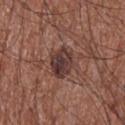<record>
  <biopsy_status>not biopsied; imaged during a skin examination</biopsy_status>
  <automated_metrics>
    <cielab_L>36</cielab_L>
    <cielab_a>19</cielab_a>
    <cielab_b>21</cielab_b>
    <vs_skin_darker_L>11.0</vs_skin_darker_L>
    <vs_skin_contrast_norm>10.0</vs_skin_contrast_norm>
    <lesion_detection_confidence_0_100>100</lesion_detection_confidence_0_100>
  </automated_metrics>
  <patient>
    <sex>male</sex>
    <age_approx>75</age_approx>
  </patient>
  <lighting>white-light</lighting>
  <lesion_size>
    <long_diameter_mm_approx>4.0</long_diameter_mm_approx>
  </lesion_size>
  <image>
    <source>total-body photography crop</source>
    <field_of_view_mm>15</field_of_view_mm>
  </image>
  <site>front of the torso</site>
</record>